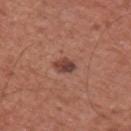Notes:
– tile lighting: white-light
– acquisition: ~15 mm crop, total-body skin-cancer survey
– site: the left upper arm
– lesion size: ≈3 mm
– patient: male, roughly 45 years of age